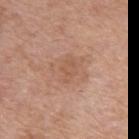Findings:
* notes — imaged on a skin check; not biopsied
* image — total-body-photography crop, ~15 mm field of view
* body site — the left upper arm
* size — ≈3 mm
* lighting — white-light illumination
* subject — male, in their mid- to late 50s